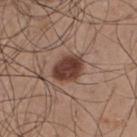No biopsy was performed on this lesion — it was imaged during a full skin examination and was not determined to be concerning. A male patient about 50 years old. From the upper back. A 15 mm close-up extracted from a 3D total-body photography capture.